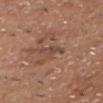biopsy status — total-body-photography surveillance lesion; no biopsy
image-analysis metrics — a lesion area of about 5 mm², an outline eccentricity of about 0.85 (0 = round, 1 = elongated), and a symmetry-axis asymmetry near 0.4; an average lesion color of about L≈46 a*≈20 b*≈27 (CIELAB), about 7 CIELAB-L* units darker than the surrounding skin, and a normalized lesion–skin contrast near 5.5; a border-irregularity rating of about 5.5/10, a within-lesion color-variation index near 3/10, and radial color variation of about 1; a lesion-detection confidence of about 90/100
lighting — white-light illumination
size — ~3.5 mm (longest diameter)
subject — male, roughly 70 years of age
body site — the chest
image — 15 mm crop, total-body photography